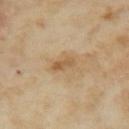Part of a total-body skin-imaging series; this lesion was reviewed on a skin check and was not flagged for biopsy. Located on the arm. A female patient, aged around 35. The lesion-visualizer software estimated an outline eccentricity of about 0.85 (0 = round, 1 = elongated) and a symmetry-axis asymmetry near 0.3. And it measured a mean CIELAB color near L≈54 a*≈15 b*≈35, roughly 9 lightness units darker than nearby skin, and a normalized lesion–skin contrast near 6.5. It also reported a within-lesion color-variation index near 3/10. The analysis additionally found an automated nevus-likeness rating near 0 out of 100. A 15 mm close-up tile from a total-body photography series done for melanoma screening. Longest diameter approximately 3 mm. Imaged with cross-polarized lighting.